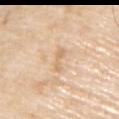workup: catalogued during a skin exam; not biopsied
patient: male, approximately 80 years of age
location: the upper back
image source: total-body-photography crop, ~15 mm field of view
lesion diameter: about 3 mm
lighting: white-light illumination